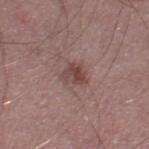Captured during whole-body skin photography for melanoma surveillance; the lesion was not biopsied.